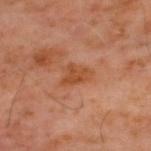Q: Was a biopsy performed?
A: total-body-photography surveillance lesion; no biopsy
Q: What lighting was used for the tile?
A: cross-polarized
Q: What is the imaging modality?
A: ~15 mm tile from a whole-body skin photo
Q: What are the patient's age and sex?
A: male, in their 60s
Q: Lesion location?
A: the upper back
Q: Lesion size?
A: ≈3.5 mm